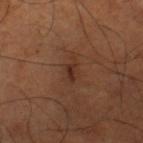{
  "biopsy_status": "not biopsied; imaged during a skin examination",
  "site": "right thigh",
  "image": {
    "source": "total-body photography crop",
    "field_of_view_mm": 15
  },
  "patient": {
    "sex": "male",
    "age_approx": 65
  },
  "lesion_size": {
    "long_diameter_mm_approx": 3.0
  },
  "lighting": "cross-polarized",
  "automated_metrics": {
    "cielab_L": 31,
    "cielab_a": 20,
    "cielab_b": 27,
    "vs_skin_darker_L": 7.0,
    "vs_skin_contrast_norm": 7.0,
    "border_irregularity_0_10": 3.5,
    "color_variation_0_10": 2.5,
    "peripheral_color_asymmetry": 1.0
  }
}